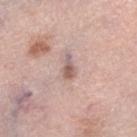The lesion was photographed on a routine skin check and not biopsied; there is no pathology result. Automated tile analysis of the lesion measured border irregularity of about 5 on a 0–10 scale and radial color variation of about 0.5. It also reported an automated nevus-likeness rating near 0 out of 100 and a lesion-detection confidence of about 90/100. A female patient, aged 63–67. From the right lower leg. Cropped from a total-body skin-imaging series; the visible field is about 15 mm. Captured under white-light illumination. About 3 mm across.